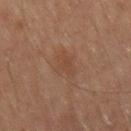This lesion was catalogued during total-body skin photography and was not selected for biopsy.
Measured at roughly 3 mm in maximum diameter.
Automated image analysis of the tile measured radial color variation of about 0.5. The software also gave a detector confidence of about 100 out of 100 that the crop contains a lesion.
A region of skin cropped from a whole-body photographic capture, roughly 15 mm wide.
This is a cross-polarized tile.
A male patient in their 70s.
The lesion is located on the right thigh.